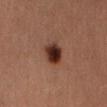| feature | finding |
|---|---|
| illumination | cross-polarized |
| patient | female, roughly 30 years of age |
| lesion size | ~3 mm (longest diameter) |
| anatomic site | the abdomen |
| TBP lesion metrics | a lesion area of about 7 mm², a shape eccentricity near 0.4, and a symmetry-axis asymmetry near 0.15; border irregularity of about 1.5 on a 0–10 scale, a color-variation rating of about 5.5/10, and peripheral color asymmetry of about 1.5 |
| image source | ~15 mm tile from a whole-body skin photo |